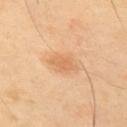Clinical impression:
Captured during whole-body skin photography for melanoma surveillance; the lesion was not biopsied.
Background:
Measured at roughly 3.5 mm in maximum diameter. A male patient approximately 65 years of age. This is a cross-polarized tile. The total-body-photography lesion software estimated border irregularity of about 2.5 on a 0–10 scale, a color-variation rating of about 1.5/10, and radial color variation of about 0.5. And it measured a nevus-likeness score of about 30/100. A 15 mm close-up tile from a total-body photography series done for melanoma screening. On the upper back.